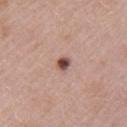This is a white-light tile. A female subject, roughly 30 years of age. Located on the left upper arm. Cropped from a whole-body photographic skin survey; the tile spans about 15 mm. Automated image analysis of the tile measured an average lesion color of about L≈48 a*≈21 b*≈23 (CIELAB), about 17 CIELAB-L* units darker than the surrounding skin, and a normalized border contrast of about 11.5. The software also gave a classifier nevus-likeness of about 100/100. Histopathologically confirmed as an atypical melanocytic neoplasm (borderline).